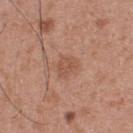Clinical summary: The recorded lesion diameter is about 2.5 mm. This is a white-light tile. A roughly 15 mm field-of-view crop from a total-body skin photograph. The lesion is located on the upper back. A male patient aged around 30. The lesion-visualizer software estimated a mean CIELAB color near L≈53 a*≈22 b*≈30, roughly 7 lightness units darker than nearby skin, and a lesion-to-skin contrast of about 5 (normalized; higher = more distinct). The analysis additionally found a border-irregularity rating of about 3/10 and a within-lesion color-variation index near 1.5/10. And it measured lesion-presence confidence of about 100/100.